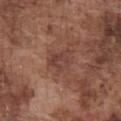follow-up: no biopsy performed (imaged during a skin exam)
automated metrics: a lesion–skin lightness drop of about 7 and a normalized lesion–skin contrast near 6; border irregularity of about 3.5 on a 0–10 scale and peripheral color asymmetry of about 1.5; an automated nevus-likeness rating near 0 out of 100 and a detector confidence of about 100 out of 100 that the crop contains a lesion
lesion diameter: ≈3.5 mm
lighting: white-light illumination
subject: male, approximately 75 years of age
body site: the chest
image: 15 mm crop, total-body photography